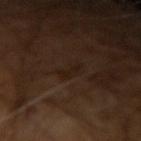notes — total-body-photography surveillance lesion; no biopsy
subject — male, roughly 65 years of age
image source — 15 mm crop, total-body photography